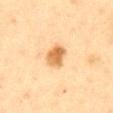Notes:
• lesion diameter: about 3 mm
• patient: female, approximately 55 years of age
• imaging modality: total-body-photography crop, ~15 mm field of view
• body site: the abdomen